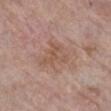Q: Was a biopsy performed?
A: imaged on a skin check; not biopsied
Q: What are the patient's age and sex?
A: female, approximately 70 years of age
Q: Automated lesion metrics?
A: an average lesion color of about L≈53 a*≈19 b*≈28 (CIELAB), a lesion–skin lightness drop of about 6, and a normalized lesion–skin contrast near 5.5; border irregularity of about 7 on a 0–10 scale and radial color variation of about 1
Q: Lesion size?
A: about 4 mm
Q: Where on the body is the lesion?
A: the right lower leg
Q: How was this image acquired?
A: ~15 mm tile from a whole-body skin photo